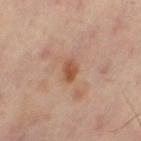workup=no biopsy performed (imaged during a skin exam) | patient=female, aged 38–42 | imaging modality=~15 mm tile from a whole-body skin photo | lesion size=about 2.5 mm | lighting=cross-polarized | location=the left thigh.